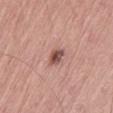Part of a total-body skin-imaging series; this lesion was reviewed on a skin check and was not flagged for biopsy. Automated tile analysis of the lesion measured lesion-presence confidence of about 100/100. A male patient aged approximately 80. A region of skin cropped from a whole-body photographic capture, roughly 15 mm wide. Captured under white-light illumination. Located on the left thigh.